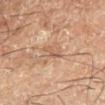follow-up: catalogued during a skin exam; not biopsied | image: 15 mm crop, total-body photography | patient: male, aged 63 to 67 | anatomic site: the leg.